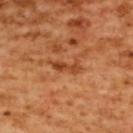Background:
Measured at roughly 4 mm in maximum diameter. A lesion tile, about 15 mm wide, cut from a 3D total-body photograph. From the upper back. The patient is a female in their mid-50s. Imaged with cross-polarized lighting. The lesion-visualizer software estimated an area of roughly 4.5 mm² and a shape-asymmetry score of about 0.4 (0 = symmetric). And it measured a lesion color around L≈48 a*≈29 b*≈41 in CIELAB and about 9 CIELAB-L* units darker than the surrounding skin. The analysis additionally found internal color variation of about 2.5 on a 0–10 scale and radial color variation of about 0.5. It also reported an automated nevus-likeness rating near 0 out of 100 and lesion-presence confidence of about 100/100.